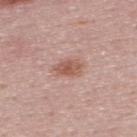Clinical impression: Recorded during total-body skin imaging; not selected for excision or biopsy.